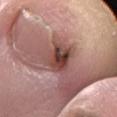The lesion was photographed on a routine skin check and not biopsied; there is no pathology result. Automated image analysis of the tile measured a lesion area of about 10 mm². It also reported a mean CIELAB color near L≈44 a*≈22 b*≈23. The analysis additionally found border irregularity of about 3 on a 0–10 scale, internal color variation of about 9 on a 0–10 scale, and radial color variation of about 3. The tile uses white-light illumination. From the left lower leg. A region of skin cropped from a whole-body photographic capture, roughly 15 mm wide. Approximately 4 mm at its widest. A male patient, aged around 60.